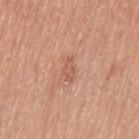Imaged during a routine full-body skin examination; the lesion was not biopsied and no histopathology is available.
From the left thigh.
The total-body-photography lesion software estimated an area of roughly 3 mm², an outline eccentricity of about 0.85 (0 = round, 1 = elongated), and a shape-asymmetry score of about 0.4 (0 = symmetric). It also reported a border-irregularity index near 4/10 and radial color variation of about 0.5. The software also gave an automated nevus-likeness rating near 0 out of 100.
A close-up tile cropped from a whole-body skin photograph, about 15 mm across.
The patient is a female approximately 55 years of age.
About 2.5 mm across.